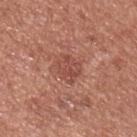Case summary:
* follow-up: catalogued during a skin exam; not biopsied
* lesion diameter: ~3.5 mm (longest diameter)
* body site: the upper back
* lighting: white-light illumination
* automated metrics: an area of roughly 6.5 mm², a shape eccentricity near 0.55, and two-axis asymmetry of about 0.2; a border-irregularity rating of about 2.5/10, a within-lesion color-variation index near 3/10, and peripheral color asymmetry of about 1
* subject: male, aged 53 to 57
* acquisition: 15 mm crop, total-body photography